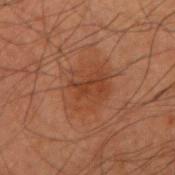Assessment: Captured during whole-body skin photography for melanoma surveillance; the lesion was not biopsied. Image and clinical context: Cropped from a whole-body photographic skin survey; the tile spans about 15 mm. From the right upper arm. A male patient aged 58 to 62.